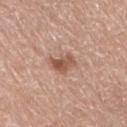Captured during whole-body skin photography for melanoma surveillance; the lesion was not biopsied. Captured under white-light illumination. The total-body-photography lesion software estimated an outline eccentricity of about 0.7 (0 = round, 1 = elongated). It also reported an automated nevus-likeness rating near 55 out of 100 and lesion-presence confidence of about 100/100. This image is a 15 mm lesion crop taken from a total-body photograph. The patient is a female approximately 75 years of age. From the right thigh.